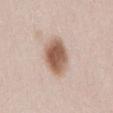Q: Was this lesion biopsied?
A: imaged on a skin check; not biopsied
Q: What did automated image analysis measure?
A: a footprint of about 12 mm²; roughly 16 lightness units darker than nearby skin; a nevus-likeness score of about 100/100
Q: What is the anatomic site?
A: the lower back
Q: What kind of image is this?
A: ~15 mm tile from a whole-body skin photo
Q: What are the patient's age and sex?
A: female, in their 20s
Q: How large is the lesion?
A: ≈4.5 mm